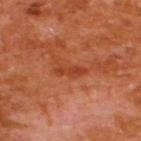No biopsy was performed on this lesion — it was imaged during a full skin examination and was not determined to be concerning. The total-body-photography lesion software estimated a footprint of about 4 mm², an eccentricity of roughly 0.95, and a shape-asymmetry score of about 0.35 (0 = symmetric). The software also gave an average lesion color of about L≈41 a*≈31 b*≈37 (CIELAB) and a lesion-to-skin contrast of about 6 (normalized; higher = more distinct). The analysis additionally found border irregularity of about 4 on a 0–10 scale and radial color variation of about 0. The software also gave a classifier nevus-likeness of about 0/100. The subject is a male aged 63–67. Captured under cross-polarized illumination. The lesion is located on the upper back. Longest diameter approximately 3.5 mm. A 15 mm close-up extracted from a 3D total-body photography capture.